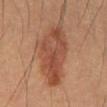The lesion was tiled from a total-body skin photograph and was not biopsied.
The lesion is on the mid back.
About 9.5 mm across.
The patient is a male aged approximately 55.
Cropped from a total-body skin-imaging series; the visible field is about 15 mm.
Captured under cross-polarized illumination.